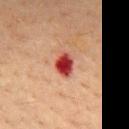Impression:
The lesion was tiled from a total-body skin photograph and was not biopsied.
Acquisition and patient details:
A region of skin cropped from a whole-body photographic capture, roughly 15 mm wide. A female patient, roughly 55 years of age. Located on the chest.